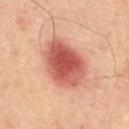This lesion was catalogued during total-body skin photography and was not selected for biopsy.
The lesion is on the chest.
A male patient, aged approximately 65.
The tile uses cross-polarized illumination.
Cropped from a total-body skin-imaging series; the visible field is about 15 mm.
Automated image analysis of the tile measured a lesion–skin lightness drop of about 13. The analysis additionally found border irregularity of about 1.5 on a 0–10 scale, internal color variation of about 5 on a 0–10 scale, and a peripheral color-asymmetry measure near 1.5.
The lesion's longest dimension is about 6.5 mm.